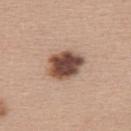<lesion>
<biopsy_status>not biopsied; imaged during a skin examination</biopsy_status>
<image>
  <source>total-body photography crop</source>
  <field_of_view_mm>15</field_of_view_mm>
</image>
<lesion_size>
  <long_diameter_mm_approx>4.5</long_diameter_mm_approx>
</lesion_size>
<automated_metrics>
  <cielab_L>47</cielab_L>
  <cielab_a>20</cielab_a>
  <cielab_b>26</cielab_b>
  <vs_skin_darker_L>20.0</vs_skin_darker_L>
  <vs_skin_contrast_norm>13.5</vs_skin_contrast_norm>
  <color_variation_0_10>5.5</color_variation_0_10>
  <peripheral_color_asymmetry>1.5</peripheral_color_asymmetry>
  <nevus_likeness_0_100>85</nevus_likeness_0_100>
  <lesion_detection_confidence_0_100>100</lesion_detection_confidence_0_100>
</automated_metrics>
<lighting>white-light</lighting>
<patient>
  <sex>female</sex>
  <age_approx>40</age_approx>
</patient>
<site>upper back</site>
</lesion>